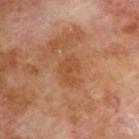Impression:
The lesion was tiled from a total-body skin photograph and was not biopsied.
Image and clinical context:
A 15 mm close-up tile from a total-body photography series done for melanoma screening. On the upper back. A male subject, aged 68–72. The lesion's longest dimension is about 3 mm.